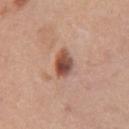<tbp_lesion>
<biopsy_status>not biopsied; imaged during a skin examination</biopsy_status>
<site>right upper arm</site>
<patient>
  <sex>female</sex>
  <age_approx>60</age_approx>
</patient>
<lesion_size>
  <long_diameter_mm_approx>3.0</long_diameter_mm_approx>
</lesion_size>
<image>
  <source>total-body photography crop</source>
  <field_of_view_mm>15</field_of_view_mm>
</image>
<lighting>white-light</lighting>
</tbp_lesion>